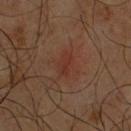follow-up: imaged on a skin check; not biopsied
lesion diameter: ≈2.5 mm
subject: male, aged 58–62
lighting: cross-polarized
site: the chest
acquisition: ~15 mm tile from a whole-body skin photo